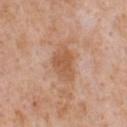Part of a total-body skin-imaging series; this lesion was reviewed on a skin check and was not flagged for biopsy. Cropped from a whole-body photographic skin survey; the tile spans about 15 mm. Longest diameter approximately 4.5 mm. From the chest. A male subject, about 65 years old. Automated image analysis of the tile measured a mean CIELAB color near L≈57 a*≈22 b*≈34, roughly 9 lightness units darker than nearby skin, and a normalized lesion–skin contrast near 7. It also reported a within-lesion color-variation index near 2.5/10. And it measured lesion-presence confidence of about 100/100.